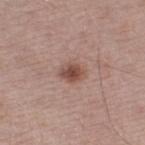biopsy status: catalogued during a skin exam; not biopsied | site: the leg | image: total-body-photography crop, ~15 mm field of view | patient: male, roughly 55 years of age.